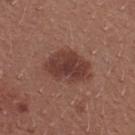biopsy status — total-body-photography surveillance lesion; no biopsy
acquisition — total-body-photography crop, ~15 mm field of view
site — the upper back
lesion size — about 5.5 mm
subject — female, aged 23 to 27
automated lesion analysis — a border-irregularity rating of about 2/10
lighting — white-light